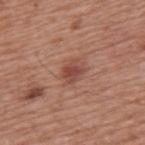The lesion was photographed on a routine skin check and not biopsied; there is no pathology result. Cropped from a whole-body photographic skin survey; the tile spans about 15 mm. The lesion is on the back. The patient is a male aged 73 to 77.